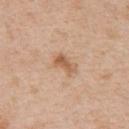<lesion>
<biopsy_status>not biopsied; imaged during a skin examination</biopsy_status>
<image>
  <source>total-body photography crop</source>
  <field_of_view_mm>15</field_of_view_mm>
</image>
<site>chest</site>
<patient>
  <sex>female</sex>
  <age_approx>40</age_approx>
</patient>
</lesion>